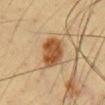No biopsy was performed on this lesion — it was imaged during a full skin examination and was not determined to be concerning. The lesion's longest dimension is about 4.5 mm. The patient is a male in their mid- to late 40s. Cropped from a whole-body photographic skin survey; the tile spans about 15 mm. On the chest. The tile uses cross-polarized illumination. The total-body-photography lesion software estimated a footprint of about 11 mm², a shape eccentricity near 0.75, and a shape-asymmetry score of about 0.15 (0 = symmetric). It also reported a border-irregularity index near 1.5/10, a color-variation rating of about 4.5/10, and peripheral color asymmetry of about 1.5. The software also gave a nevus-likeness score of about 100/100 and lesion-presence confidence of about 100/100.